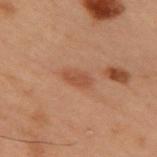• follow-up — catalogued during a skin exam; not biopsied
• image — ~15 mm crop, total-body skin-cancer survey
• lesion diameter — ≈3 mm
• tile lighting — cross-polarized illumination
• image-analysis metrics — a mean CIELAB color near L≈40 a*≈20 b*≈28, roughly 7 lightness units darker than nearby skin, and a lesion-to-skin contrast of about 6 (normalized; higher = more distinct); a color-variation rating of about 1.5/10 and radial color variation of about 0.5
• patient — male, roughly 55 years of age
• anatomic site — the upper back